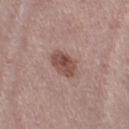Notes:
* biopsy status — total-body-photography surveillance lesion; no biopsy
* location — the right thigh
* illumination — white-light illumination
* image — 15 mm crop, total-body photography
* patient — female, in their 40s
* lesion size — ~4 mm (longest diameter)